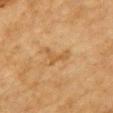This lesion was catalogued during total-body skin photography and was not selected for biopsy.
The patient is a male aged approximately 85 or older.
Automated tile analysis of the lesion measured an area of roughly 4.5 mm², an outline eccentricity of about 0.85 (0 = round, 1 = elongated), and two-axis asymmetry of about 0.65. The software also gave internal color variation of about 1 on a 0–10 scale.
A roughly 15 mm field-of-view crop from a total-body skin photograph.
The lesion is on the chest.
Measured at roughly 3.5 mm in maximum diameter.